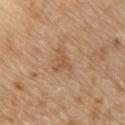workup = imaged on a skin check; not biopsied | subject = male, about 70 years old | anatomic site = the chest | image = ~15 mm crop, total-body skin-cancer survey.